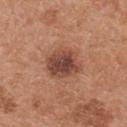A close-up tile cropped from a whole-body skin photograph, about 15 mm across.
Approximately 4.5 mm at its widest.
Located on the upper back.
Automated image analysis of the tile measured a mean CIELAB color near L≈46 a*≈24 b*≈28 and a normalized lesion–skin contrast near 9.5.
A female patient, about 40 years old.